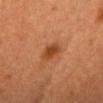{"biopsy_status": "not biopsied; imaged during a skin examination", "site": "head or neck", "lesion_size": {"long_diameter_mm_approx": 3.5}, "lighting": "cross-polarized", "image": {"source": "total-body photography crop", "field_of_view_mm": 15}, "patient": {"sex": "female", "age_approx": 40}}